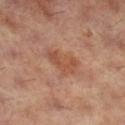| field | value |
|---|---|
| follow-up | catalogued during a skin exam; not biopsied |
| imaging modality | ~15 mm tile from a whole-body skin photo |
| lighting | cross-polarized |
| patient | female, aged around 60 |
| lesion size | ≈4 mm |
| TBP lesion metrics | a footprint of about 8 mm², an eccentricity of roughly 0.8, and a shape-asymmetry score of about 0.4 (0 = symmetric); a border-irregularity rating of about 4.5/10, a color-variation rating of about 2.5/10, and peripheral color asymmetry of about 0.5 |
| site | the right lower leg |